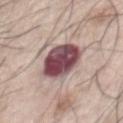No biopsy was performed on this lesion — it was imaged during a full skin examination and was not determined to be concerning. Located on the chest. The recorded lesion diameter is about 5 mm. A male subject in their 70s. A lesion tile, about 15 mm wide, cut from a 3D total-body photograph. The tile uses white-light illumination.